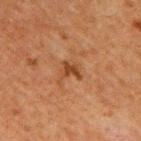follow-up = total-body-photography surveillance lesion; no biopsy | image source = ~15 mm tile from a whole-body skin photo | body site = the back | patient = male, in their 60s | lighting = cross-polarized illumination | lesion size = about 2.5 mm.